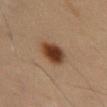{
  "biopsy_status": "not biopsied; imaged during a skin examination",
  "lesion_size": {
    "long_diameter_mm_approx": 3.5
  },
  "site": "abdomen",
  "patient": {
    "sex": "male",
    "age_approx": 55
  },
  "image": {
    "source": "total-body photography crop",
    "field_of_view_mm": 15
  },
  "automated_metrics": {
    "area_mm2_approx": 8.0,
    "eccentricity": 0.5,
    "cielab_L": 38,
    "cielab_a": 20,
    "cielab_b": 30,
    "vs_skin_darker_L": 16.0,
    "vs_skin_contrast_norm": 12.5
  }
}